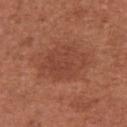{
  "biopsy_status": "not biopsied; imaged during a skin examination",
  "image": {
    "source": "total-body photography crop",
    "field_of_view_mm": 15
  },
  "site": "arm",
  "patient": {
    "sex": "female",
    "age_approx": 65
  }
}